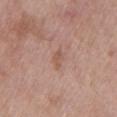Assessment:
Imaged during a routine full-body skin examination; the lesion was not biopsied and no histopathology is available.
Image and clinical context:
A male subject, aged approximately 60. A 15 mm crop from a total-body photograph taken for skin-cancer surveillance. Captured under white-light illumination. About 2.5 mm across. The lesion is on the chest.